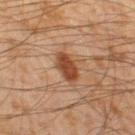tile lighting — cross-polarized illumination
patient — male, approximately 45 years of age
location — the left thigh
TBP lesion metrics — an area of roughly 6.5 mm², an outline eccentricity of about 0.75 (0 = round, 1 = elongated), and a shape-asymmetry score of about 0.2 (0 = symmetric); a mean CIELAB color near L≈37 a*≈20 b*≈28, a lesion–skin lightness drop of about 11, and a lesion-to-skin contrast of about 9.5 (normalized; higher = more distinct); a border-irregularity index near 2/10, internal color variation of about 2.5 on a 0–10 scale, and radial color variation of about 1; an automated nevus-likeness rating near 95 out of 100 and a lesion-detection confidence of about 100/100
image — ~15 mm tile from a whole-body skin photo